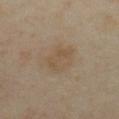follow-up: imaged on a skin check; not biopsied | subject: female, roughly 40 years of age | anatomic site: the chest | imaging modality: total-body-photography crop, ~15 mm field of view.